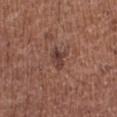biopsy status: no biopsy performed (imaged during a skin exam); anatomic site: the right lower leg; image source: 15 mm crop, total-body photography; patient: female, aged approximately 50.